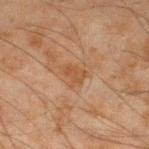follow-up = catalogued during a skin exam; not biopsied | imaging modality = total-body-photography crop, ~15 mm field of view | automated metrics = a border-irregularity index near 4/10, a within-lesion color-variation index near 3/10, and peripheral color asymmetry of about 1; a nevus-likeness score of about 0/100 and lesion-presence confidence of about 100/100 | lesion size = ~3 mm (longest diameter) | body site = the left lower leg | patient = male, in their mid-40s | illumination = cross-polarized illumination.